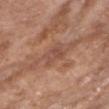Q: Is there a histopathology result?
A: total-body-photography surveillance lesion; no biopsy
Q: How was this image acquired?
A: ~15 mm tile from a whole-body skin photo
Q: What did automated image analysis measure?
A: a lesion–skin lightness drop of about 7 and a normalized border contrast of about 5.5; a border-irregularity rating of about 2.5/10 and internal color variation of about 3.5 on a 0–10 scale; lesion-presence confidence of about 100/100
Q: Patient demographics?
A: female, about 75 years old
Q: How large is the lesion?
A: ~2.5 mm (longest diameter)
Q: Illumination type?
A: white-light illumination
Q: What is the anatomic site?
A: the chest